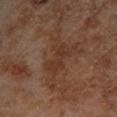Captured during whole-body skin photography for melanoma surveillance; the lesion was not biopsied. The lesion is on the right lower leg. A male patient, aged 68–72. The total-body-photography lesion software estimated a lesion color around L≈33 a*≈19 b*≈26 in CIELAB and roughly 6 lightness units darker than nearby skin. The software also gave internal color variation of about 2 on a 0–10 scale and a peripheral color-asymmetry measure near 1. A close-up tile cropped from a whole-body skin photograph, about 15 mm across. About 4 mm across. This is a cross-polarized tile.